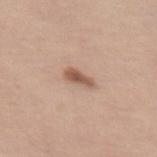workup: total-body-photography surveillance lesion; no biopsy | illumination: white-light illumination | site: the leg | patient: female, aged approximately 55 | image: 15 mm crop, total-body photography | lesion diameter: ~3 mm (longest diameter).